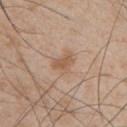workup = catalogued during a skin exam; not biopsied
patient = male, about 50 years old
site = the chest
image source = ~15 mm tile from a whole-body skin photo
size = ≈3 mm
tile lighting = white-light